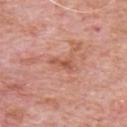follow-up = no biopsy performed (imaged during a skin exam) | site = the chest | imaging modality = ~15 mm tile from a whole-body skin photo | subject = male, about 80 years old | automated lesion analysis = a lesion area of about 3 mm² and a symmetry-axis asymmetry near 0.5; an automated nevus-likeness rating near 0 out of 100 and a lesion-detection confidence of about 100/100 | lesion size = about 3 mm.